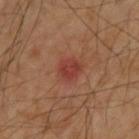workup = total-body-photography surveillance lesion; no biopsy
automated metrics = a lesion color around L≈38 a*≈28 b*≈28 in CIELAB, a lesion–skin lightness drop of about 8, and a normalized border contrast of about 7; border irregularity of about 2 on a 0–10 scale and internal color variation of about 4 on a 0–10 scale; lesion-presence confidence of about 100/100
image = ~15 mm tile from a whole-body skin photo
subject = male, in their mid- to late 50s
illumination = cross-polarized
site = the upper back